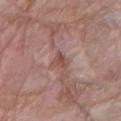Recorded during total-body skin imaging; not selected for excision or biopsy.
Longest diameter approximately 2.5 mm.
Located on the right forearm.
The lesion-visualizer software estimated a shape eccentricity near 0.8. The software also gave a mean CIELAB color near L≈49 a*≈20 b*≈24, about 9 CIELAB-L* units darker than the surrounding skin, and a lesion-to-skin contrast of about 7 (normalized; higher = more distinct). It also reported a classifier nevus-likeness of about 0/100 and a detector confidence of about 85 out of 100 that the crop contains a lesion.
A female subject, approximately 65 years of age.
A close-up tile cropped from a whole-body skin photograph, about 15 mm across.
Imaged with white-light lighting.